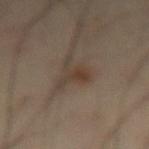follow-up: imaged on a skin check; not biopsied
body site: the right thigh
lesion size: ~4.5 mm (longest diameter)
subject: male, aged 53 to 57
image source: 15 mm crop, total-body photography
tile lighting: cross-polarized illumination